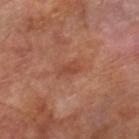Captured during whole-body skin photography for melanoma surveillance; the lesion was not biopsied.